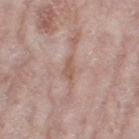Imaged during a routine full-body skin examination; the lesion was not biopsied and no histopathology is available. Automated tile analysis of the lesion measured a classifier nevus-likeness of about 0/100. This is a white-light tile. A region of skin cropped from a whole-body photographic capture, roughly 15 mm wide. The subject is a female aged 68 to 72. Longest diameter approximately 3 mm. Located on the right thigh.